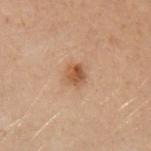<record>
  <site>left forearm</site>
  <image>
    <source>total-body photography crop</source>
    <field_of_view_mm>15</field_of_view_mm>
  </image>
  <patient>
    <sex>female</sex>
    <age_approx>30</age_approx>
  </patient>
  <lesion_size>
    <long_diameter_mm_approx>2.5</long_diameter_mm_approx>
  </lesion_size>
</record>